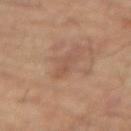Imaged during a routine full-body skin examination; the lesion was not biopsied and no histopathology is available.
This is a cross-polarized tile.
On the right forearm.
The lesion's longest dimension is about 3.5 mm.
A close-up tile cropped from a whole-body skin photograph, about 15 mm across.
The lesion-visualizer software estimated a footprint of about 3.5 mm², a shape eccentricity near 0.95, and a shape-asymmetry score of about 0.3 (0 = symmetric). And it measured a mean CIELAB color near L≈53 a*≈19 b*≈30.
A male patient, aged 53 to 57.